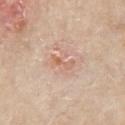The lesion was tiled from a total-body skin photograph and was not biopsied.
Cropped from a total-body skin-imaging series; the visible field is about 15 mm.
This is a white-light tile.
The patient is a female in their 70s.
The lesion is located on the chest.